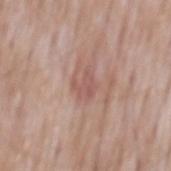Part of a total-body skin-imaging series; this lesion was reviewed on a skin check and was not flagged for biopsy. Located on the mid back. Measured at roughly 2.5 mm in maximum diameter. A male patient, aged approximately 65. The total-body-photography lesion software estimated a classifier nevus-likeness of about 0/100 and lesion-presence confidence of about 100/100. A 15 mm close-up extracted from a 3D total-body photography capture. The tile uses white-light illumination.